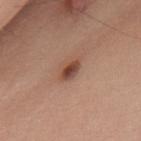Case summary:
– notes · imaged on a skin check; not biopsied
– subject · male, about 50 years old
– image · ~15 mm crop, total-body skin-cancer survey
– lesion size · ≈3 mm
– tile lighting · white-light
– location · the upper back
– TBP lesion metrics · a lesion color around L≈46 a*≈23 b*≈29 in CIELAB, about 13 CIELAB-L* units darker than the surrounding skin, and a lesion-to-skin contrast of about 9.5 (normalized; higher = more distinct); border irregularity of about 2.5 on a 0–10 scale and internal color variation of about 6 on a 0–10 scale; an automated nevus-likeness rating near 95 out of 100